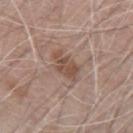* biopsy status — total-body-photography surveillance lesion; no biopsy
* automated lesion analysis — a symmetry-axis asymmetry near 0.3; an average lesion color of about L≈50 a*≈18 b*≈25 (CIELAB) and a lesion-to-skin contrast of about 7 (normalized; higher = more distinct); a color-variation rating of about 2.5/10 and peripheral color asymmetry of about 0.5
* image source — ~15 mm crop, total-body skin-cancer survey
* site — the left upper arm
* size — ~4 mm (longest diameter)
* subject — male, aged 68–72